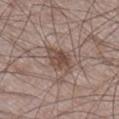Part of a total-body skin-imaging series; this lesion was reviewed on a skin check and was not flagged for biopsy.
A close-up tile cropped from a whole-body skin photograph, about 15 mm across.
About 4 mm across.
A male subject, about 60 years old.
This is a white-light tile.
Located on the leg.